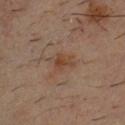Q: Was a biopsy performed?
A: no biopsy performed (imaged during a skin exam)
Q: What are the patient's age and sex?
A: male, aged around 35
Q: Where on the body is the lesion?
A: the chest
Q: Automated lesion metrics?
A: a footprint of about 5.5 mm², an eccentricity of roughly 0.7, and a symmetry-axis asymmetry near 0.35; a nevus-likeness score of about 5/100
Q: How was this image acquired?
A: ~15 mm crop, total-body skin-cancer survey